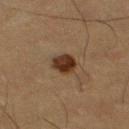Recorded during total-body skin imaging; not selected for excision or biopsy.
A region of skin cropped from a whole-body photographic capture, roughly 15 mm wide.
Measured at roughly 3 mm in maximum diameter.
A male subject aged 58–62.
The lesion-visualizer software estimated a lesion area of about 6.5 mm², an outline eccentricity of about 0.35 (0 = round, 1 = elongated), and a symmetry-axis asymmetry near 0.2. The analysis additionally found a nevus-likeness score of about 95/100.
The lesion is on the left thigh.
This is a cross-polarized tile.